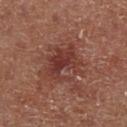workup: total-body-photography surveillance lesion; no biopsy, image source: ~15 mm tile from a whole-body skin photo, illumination: cross-polarized, anatomic site: the left lower leg, size: ≈5 mm, patient: female.